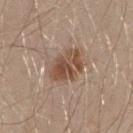Q: Was this lesion biopsied?
A: no biopsy performed (imaged during a skin exam)
Q: Patient demographics?
A: male, aged 28 to 32
Q: What is the imaging modality?
A: ~15 mm crop, total-body skin-cancer survey
Q: Where on the body is the lesion?
A: the mid back
Q: What lighting was used for the tile?
A: white-light